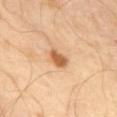Findings:
* follow-up — catalogued during a skin exam; not biopsied
* acquisition — ~15 mm tile from a whole-body skin photo
* size — ~3 mm (longest diameter)
* automated lesion analysis — an average lesion color of about L≈59 a*≈23 b*≈39 (CIELAB), roughly 14 lightness units darker than nearby skin, and a normalized lesion–skin contrast near 9; a border-irregularity index near 2.5/10, a within-lesion color-variation index near 1.5/10, and a peripheral color-asymmetry measure near 0.5
* subject — male, aged approximately 65
* illumination — cross-polarized